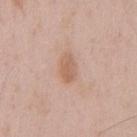Q: Was this lesion biopsied?
A: imaged on a skin check; not biopsied
Q: Automated lesion metrics?
A: a lesion area of about 5.5 mm², an outline eccentricity of about 0.85 (0 = round, 1 = elongated), and two-axis asymmetry of about 0.35; lesion-presence confidence of about 100/100
Q: What is the imaging modality?
A: total-body-photography crop, ~15 mm field of view
Q: Lesion location?
A: the chest
Q: What are the patient's age and sex?
A: male, aged around 50
Q: How large is the lesion?
A: ≈3.5 mm